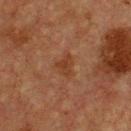No biopsy was performed on this lesion — it was imaged during a full skin examination and was not determined to be concerning.
From the chest.
Cropped from a whole-body photographic skin survey; the tile spans about 15 mm.
The tile uses cross-polarized illumination.
Approximately 2.5 mm at its widest.
The lesion-visualizer software estimated a footprint of about 4 mm² and an outline eccentricity of about 0.65 (0 = round, 1 = elongated). It also reported a color-variation rating of about 2/10 and radial color variation of about 0.5.
A male subject aged approximately 75.